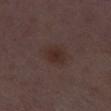This lesion was catalogued during total-body skin photography and was not selected for biopsy.
Cropped from a whole-body photographic skin survey; the tile spans about 15 mm.
The lesion is located on the right thigh.
A female patient, in their 30s.
Captured under white-light illumination.
An algorithmic analysis of the crop reported an eccentricity of roughly 0.65 and a shape-asymmetry score of about 0.25 (0 = symmetric). The analysis additionally found a border-irregularity rating of about 2/10, a within-lesion color-variation index near 2.5/10, and radial color variation of about 0.5. And it measured an automated nevus-likeness rating near 75 out of 100 and lesion-presence confidence of about 100/100.
The lesion's longest dimension is about 3 mm.